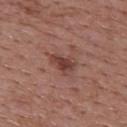This lesion was catalogued during total-body skin photography and was not selected for biopsy. Imaged with white-light lighting. About 3 mm across. The subject is a female about 50 years old. A 15 mm close-up tile from a total-body photography series done for melanoma screening. From the upper back.